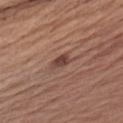Q: Was a biopsy performed?
A: total-body-photography surveillance lesion; no biopsy
Q: Automated lesion metrics?
A: an average lesion color of about L≈42 a*≈20 b*≈23 (CIELAB) and about 11 CIELAB-L* units darker than the surrounding skin; radial color variation of about 0.5; a classifier nevus-likeness of about 95/100 and a detector confidence of about 100 out of 100 that the crop contains a lesion
Q: What kind of image is this?
A: 15 mm crop, total-body photography
Q: How was the tile lit?
A: white-light
Q: Lesion location?
A: the right upper arm
Q: Lesion size?
A: ~2.5 mm (longest diameter)
Q: What are the patient's age and sex?
A: male, about 55 years old